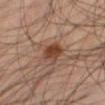workup=total-body-photography surveillance lesion; no biopsy | automated lesion analysis=an area of roughly 6 mm²; roughly 11 lightness units darker than nearby skin and a normalized lesion–skin contrast near 9.5 | body site=the right thigh | patient=male, aged around 45 | imaging modality=~15 mm tile from a whole-body skin photo | size=about 3 mm | illumination=cross-polarized illumination.